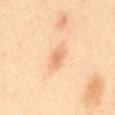workup: total-body-photography surveillance lesion; no biopsy
imaging modality: 15 mm crop, total-body photography
subject: female, roughly 40 years of age
diameter: ≈3 mm
anatomic site: the mid back